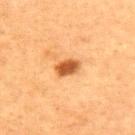lesion diameter: about 3 mm
anatomic site: the upper back
TBP lesion metrics: an area of roughly 5 mm²; a nevus-likeness score of about 100/100 and a lesion-detection confidence of about 100/100
lighting: cross-polarized illumination
patient: female, aged around 60
image source: ~15 mm crop, total-body skin-cancer survey